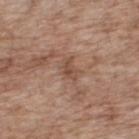No biopsy was performed on this lesion — it was imaged during a full skin examination and was not determined to be concerning.
This is a white-light tile.
Measured at roughly 2.5 mm in maximum diameter.
A 15 mm crop from a total-body photograph taken for skin-cancer surveillance.
A male subject, approximately 70 years of age.
An algorithmic analysis of the crop reported a shape eccentricity near 0.8 and a shape-asymmetry score of about 0.3 (0 = symmetric).
The lesion is on the upper back.